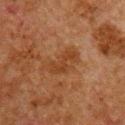Clinical impression: The lesion was photographed on a routine skin check and not biopsied; there is no pathology result. Acquisition and patient details: Cropped from a total-body skin-imaging series; the visible field is about 15 mm. Imaged with cross-polarized lighting. Located on the chest. The patient is a male about 60 years old. The recorded lesion diameter is about 4.5 mm.